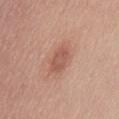Q: Was a biopsy performed?
A: no biopsy performed (imaged during a skin exam)
Q: How was this image acquired?
A: 15 mm crop, total-body photography
Q: Who is the patient?
A: male, in their mid- to late 40s
Q: Where on the body is the lesion?
A: the abdomen